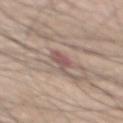biopsy_status: not biopsied; imaged during a skin examination
site: mid back
lighting: white-light
image:
  source: total-body photography crop
  field_of_view_mm: 15
patient:
  sex: male
  age_approx: 65